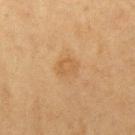  biopsy_status: not biopsied; imaged during a skin examination
  patient:
    sex: female
    age_approx: 50
  site: right upper arm
  automated_metrics:
    cielab_L: 49
    cielab_a: 17
    cielab_b: 35
    lesion_detection_confidence_0_100: 100
  image:
    source: total-body photography crop
    field_of_view_mm: 15
  lesion_size:
    long_diameter_mm_approx: 3.0
  lighting: cross-polarized